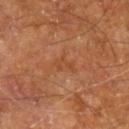patient = male, aged approximately 60
body site = the right leg
image source = ~15 mm crop, total-body skin-cancer survey